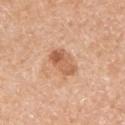Captured during whole-body skin photography for melanoma surveillance; the lesion was not biopsied. Approximately 3.5 mm at its widest. A close-up tile cropped from a whole-body skin photograph, about 15 mm across. The patient is a female aged 73–77. The lesion is on the arm. Imaged with white-light lighting.